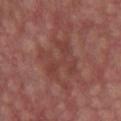* workup · total-body-photography surveillance lesion; no biopsy
* subject · male, aged 58 to 62
* lesion size · about 5 mm
* acquisition · total-body-photography crop, ~15 mm field of view
* site · the chest